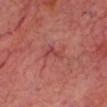notes: no biopsy performed (imaged during a skin exam) | patient: male, aged around 65 | TBP lesion metrics: a border-irregularity index near 7/10; a detector confidence of about 85 out of 100 that the crop contains a lesion | anatomic site: the head or neck | imaging modality: total-body-photography crop, ~15 mm field of view | diameter: ~3 mm (longest diameter).